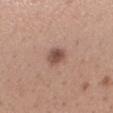The lesion was photographed on a routine skin check and not biopsied; there is no pathology result.
The lesion's longest dimension is about 2.5 mm.
A close-up tile cropped from a whole-body skin photograph, about 15 mm across.
Automated tile analysis of the lesion measured roughly 12 lightness units darker than nearby skin and a normalized lesion–skin contrast near 8.5. And it measured a border-irregularity index near 1.5/10 and peripheral color asymmetry of about 1. And it measured a classifier nevus-likeness of about 85/100.
From the arm.
A female subject, aged 28 to 32.